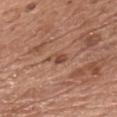follow-up: no biopsy performed (imaged during a skin exam) | location: the chest | TBP lesion metrics: a lesion–skin lightness drop of about 9; a classifier nevus-likeness of about 5/100 | lesion diameter: ~2.5 mm (longest diameter) | imaging modality: total-body-photography crop, ~15 mm field of view | illumination: white-light | patient: male, roughly 70 years of age.